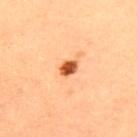{
  "biopsy_status": "not biopsied; imaged during a skin examination",
  "image": {
    "source": "total-body photography crop",
    "field_of_view_mm": 15
  },
  "automated_metrics": {
    "color_variation_0_10": 3.5
  },
  "lighting": "cross-polarized",
  "lesion_size": {
    "long_diameter_mm_approx": 2.5
  },
  "site": "upper back",
  "patient": {
    "sex": "female",
    "age_approx": 35
  }
}